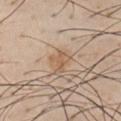Impression:
Part of a total-body skin-imaging series; this lesion was reviewed on a skin check and was not flagged for biopsy.
Context:
Automated image analysis of the tile measured a mean CIELAB color near L≈58 a*≈17 b*≈33 and a normalized border contrast of about 6.5. And it measured a border-irregularity index near 5/10, internal color variation of about 1.5 on a 0–10 scale, and peripheral color asymmetry of about 0.5. It also reported a detector confidence of about 100 out of 100 that the crop contains a lesion. The lesion is located on the front of the torso. Measured at roughly 2.5 mm in maximum diameter. Cropped from a total-body skin-imaging series; the visible field is about 15 mm. Imaged with white-light lighting. A male subject in their 60s.